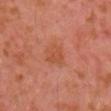The lesion was tiled from a total-body skin photograph and was not biopsied.
A close-up tile cropped from a whole-body skin photograph, about 15 mm across.
Automated tile analysis of the lesion measured a footprint of about 7 mm² and an outline eccentricity of about 0.45 (0 = round, 1 = elongated). And it measured a classifier nevus-likeness of about 0/100 and lesion-presence confidence of about 100/100.
A male subject aged approximately 30.
From the left arm.